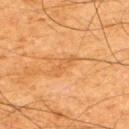| key | value |
|---|---|
| biopsy status | catalogued during a skin exam; not biopsied |
| automated lesion analysis | a footprint of about 3.5 mm², an eccentricity of roughly 0.9, and a symmetry-axis asymmetry near 0.35; a border-irregularity rating of about 4.5/10, a within-lesion color-variation index near 0/10, and peripheral color asymmetry of about 0 |
| site | the upper back |
| image | ~15 mm crop, total-body skin-cancer survey |
| patient | male, aged 63–67 |
| lighting | cross-polarized illumination |